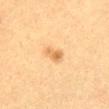The lesion was photographed on a routine skin check and not biopsied; there is no pathology result.
Cropped from a total-body skin-imaging series; the visible field is about 15 mm.
The patient is a female in their 40s.
Captured under cross-polarized illumination.
The total-body-photography lesion software estimated an average lesion color of about L≈58 a*≈17 b*≈39 (CIELAB), roughly 9 lightness units darker than nearby skin, and a normalized lesion–skin contrast near 6.5.
From the front of the torso.
Longest diameter approximately 3 mm.